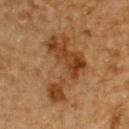Part of a total-body skin-imaging series; this lesion was reviewed on a skin check and was not flagged for biopsy. This is a cross-polarized tile. This image is a 15 mm lesion crop taken from a total-body photograph. An algorithmic analysis of the crop reported a footprint of about 23 mm² and two-axis asymmetry of about 0.65. A male patient about 85 years old or older. The lesion is located on the back. About 8 mm across.